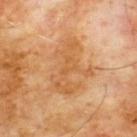Captured during whole-body skin photography for melanoma surveillance; the lesion was not biopsied. A male subject, in their 60s. The tile uses cross-polarized illumination. The total-body-photography lesion software estimated a lesion area of about 19 mm², an outline eccentricity of about 0.75 (0 = round, 1 = elongated), and a symmetry-axis asymmetry near 0.4. And it measured a border-irregularity index near 7.5/10, a color-variation rating of about 3.5/10, and peripheral color asymmetry of about 1. And it measured a classifier nevus-likeness of about 0/100 and a lesion-detection confidence of about 100/100. On the chest. A roughly 15 mm field-of-view crop from a total-body skin photograph. Approximately 6.5 mm at its widest.